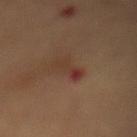{"biopsy_status": "not biopsied; imaged during a skin examination", "image": {"source": "total-body photography crop", "field_of_view_mm": 15}, "site": "mid back", "patient": {"sex": "female", "age_approx": 70}}